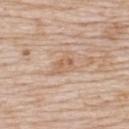notes = catalogued during a skin exam; not biopsied
acquisition = ~15 mm tile from a whole-body skin photo
anatomic site = the upper back
subject = female, approximately 70 years of age
diameter = ~3.5 mm (longest diameter)
tile lighting = white-light illumination